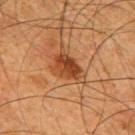| field | value |
|---|---|
| workup | catalogued during a skin exam; not biopsied |
| imaging modality | ~15 mm crop, total-body skin-cancer survey |
| subject | male, roughly 65 years of age |
| lighting | cross-polarized |
| image-analysis metrics | an average lesion color of about L≈37 a*≈22 b*≈32 (CIELAB), about 10 CIELAB-L* units darker than the surrounding skin, and a normalized lesion–skin contrast near 8.5 |
| lesion size | ≈5 mm |
| anatomic site | the upper back |